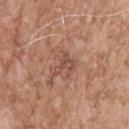{
  "biopsy_status": "not biopsied; imaged during a skin examination",
  "automated_metrics": {
    "cielab_L": 50,
    "cielab_a": 22,
    "cielab_b": 28,
    "vs_skin_darker_L": 8.0,
    "vs_skin_contrast_norm": 6.0,
    "border_irregularity_0_10": 6.0,
    "peripheral_color_asymmetry": 1.0,
    "nevus_likeness_0_100": 0
  },
  "image": {
    "source": "total-body photography crop",
    "field_of_view_mm": 15
  },
  "lesion_size": {
    "long_diameter_mm_approx": 3.0
  },
  "site": "back",
  "patient": {
    "sex": "male",
    "age_approx": 55
  },
  "lighting": "white-light"
}